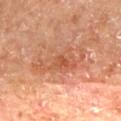{"biopsy_status": "not biopsied; imaged during a skin examination", "site": "mid back", "lesion_size": {"long_diameter_mm_approx": 6.5}, "automated_metrics": {"eccentricity": 0.9, "shape_asymmetry": 0.3, "nevus_likeness_0_100": 50}, "image": {"source": "total-body photography crop", "field_of_view_mm": 15}, "lighting": "cross-polarized", "patient": {"sex": "male", "age_approx": 65}}